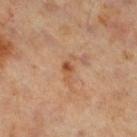Part of a total-body skin-imaging series; this lesion was reviewed on a skin check and was not flagged for biopsy. The recorded lesion diameter is about 3 mm. The patient is a male roughly 60 years of age. The tile uses cross-polarized illumination. Cropped from a whole-body photographic skin survey; the tile spans about 15 mm. From the right thigh.